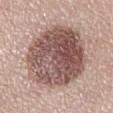No biopsy was performed on this lesion — it was imaged during a full skin examination and was not determined to be concerning.
Located on the left lower leg.
An algorithmic analysis of the crop reported an automated nevus-likeness rating near 80 out of 100 and lesion-presence confidence of about 95/100.
The tile uses white-light illumination.
The patient is a male aged approximately 50.
Measured at roughly 9 mm in maximum diameter.
A 15 mm close-up tile from a total-body photography series done for melanoma screening.